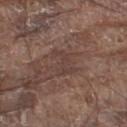* notes: total-body-photography surveillance lesion; no biopsy
* size: ≈3 mm
* imaging modality: total-body-photography crop, ~15 mm field of view
* subject: male, in their 80s
* tile lighting: white-light illumination
* automated lesion analysis: a lesion color around L≈39 a*≈17 b*≈21 in CIELAB, roughly 5 lightness units darker than nearby skin, and a normalized border contrast of about 4.5; a within-lesion color-variation index near 0/10 and a peripheral color-asymmetry measure near 0; a nevus-likeness score of about 0/100 and a lesion-detection confidence of about 80/100
* location: the left thigh